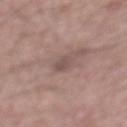Clinical impression:
This lesion was catalogued during total-body skin photography and was not selected for biopsy.
Context:
An algorithmic analysis of the crop reported an automated nevus-likeness rating near 0 out of 100 and a detector confidence of about 65 out of 100 that the crop contains a lesion. A male patient aged 53 to 57. On the mid back. A 15 mm crop from a total-body photograph taken for skin-cancer surveillance. The lesion's longest dimension is about 2.5 mm.